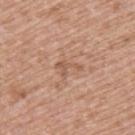{"biopsy_status": "not biopsied; imaged during a skin examination", "image": {"source": "total-body photography crop", "field_of_view_mm": 15}, "patient": {"sex": "female", "age_approx": 40}, "site": "upper back", "lesion_size": {"long_diameter_mm_approx": 3.0}, "lighting": "white-light"}